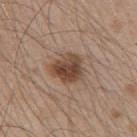The lesion was photographed on a routine skin check and not biopsied; there is no pathology result. On the back. The subject is a male about 50 years old. Imaged with white-light lighting. A 15 mm close-up tile from a total-body photography series done for melanoma screening.